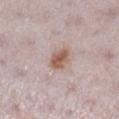<lesion>
  <biopsy_status>not biopsied; imaged during a skin examination</biopsy_status>
  <image>
    <source>total-body photography crop</source>
    <field_of_view_mm>15</field_of_view_mm>
  </image>
  <patient>
    <sex>female</sex>
    <age_approx>30</age_approx>
  </patient>
  <automated_metrics>
    <cielab_L>57</cielab_L>
    <cielab_a>19</cielab_a>
    <cielab_b>26</cielab_b>
    <vs_skin_darker_L>12.0</vs_skin_darker_L>
    <vs_skin_contrast_norm>9.0</vs_skin_contrast_norm>
    <color_variation_0_10>3.0</color_variation_0_10>
    <peripheral_color_asymmetry>1.0</peripheral_color_asymmetry>
    <lesion_detection_confidence_0_100>100</lesion_detection_confidence_0_100>
  </automated_metrics>
  <lighting>white-light</lighting>
  <site>leg</site>
</lesion>